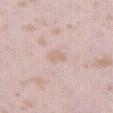{"biopsy_status": "not biopsied; imaged during a skin examination", "image": {"source": "total-body photography crop", "field_of_view_mm": 15}, "lesion_size": {"long_diameter_mm_approx": 2.5}, "lighting": "white-light", "patient": {"sex": "female", "age_approx": 25}, "automated_metrics": {"lesion_detection_confidence_0_100": 100}, "site": "right thigh"}